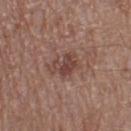follow-up: no biopsy performed (imaged during a skin exam); subject: male, aged 68–72; location: the leg; image: ~15 mm crop, total-body skin-cancer survey.